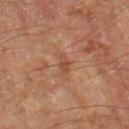{
  "biopsy_status": "not biopsied; imaged during a skin examination",
  "lesion_size": {
    "long_diameter_mm_approx": 2.5
  },
  "image": {
    "source": "total-body photography crop",
    "field_of_view_mm": 15
  },
  "automated_metrics": {
    "border_irregularity_0_10": 3.5,
    "color_variation_0_10": 1.0,
    "peripheral_color_asymmetry": 0.0
  },
  "site": "left thigh",
  "patient": {
    "sex": "male",
    "age_approx": 70
  },
  "lighting": "cross-polarized"
}